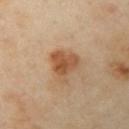{
  "biopsy_status": "not biopsied; imaged during a skin examination",
  "automated_metrics": {
    "area_mm2_approx": 9.0,
    "eccentricity": 0.4,
    "border_irregularity_0_10": 4.0,
    "color_variation_0_10": 4.0,
    "peripheral_color_asymmetry": 1.0,
    "nevus_likeness_0_100": 85,
    "lesion_detection_confidence_0_100": 100
  },
  "lesion_size": {
    "long_diameter_mm_approx": 4.0
  },
  "site": "left upper arm",
  "patient": {
    "sex": "female",
    "age_approx": 40
  },
  "lighting": "cross-polarized",
  "image": {
    "source": "total-body photography crop",
    "field_of_view_mm": 15
  }
}